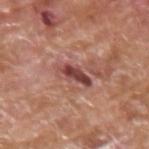{"automated_metrics": {"vs_skin_darker_L": 14.0, "vs_skin_contrast_norm": 10.5}, "image": {"source": "total-body photography crop", "field_of_view_mm": 15}, "site": "upper back", "patient": {"sex": "male", "age_approx": 65}, "lesion_size": {"long_diameter_mm_approx": 4.0}}